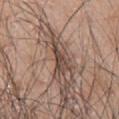Q: Was a biopsy performed?
A: total-body-photography surveillance lesion; no biopsy
Q: Who is the patient?
A: male, aged around 70
Q: How was this image acquired?
A: ~15 mm crop, total-body skin-cancer survey
Q: What lighting was used for the tile?
A: white-light illumination
Q: What is the anatomic site?
A: the left arm
Q: What is the lesion's diameter?
A: ≈3.5 mm